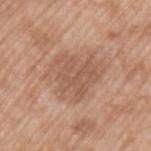Imaged during a routine full-body skin examination; the lesion was not biopsied and no histopathology is available.
This image is a 15 mm lesion crop taken from a total-body photograph.
The lesion's longest dimension is about 6 mm.
The subject is a male approximately 50 years of age.
This is a white-light tile.
The lesion is located on the left upper arm.